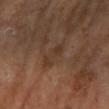  biopsy_status: not biopsied; imaged during a skin examination
  image:
    source: total-body photography crop
    field_of_view_mm: 15
  lighting: cross-polarized
  automated_metrics:
    area_mm2_approx: 4.5
    eccentricity: 0.9
    shape_asymmetry: 0.3
    cielab_L: 33
    cielab_a: 16
    cielab_b: 26
    vs_skin_darker_L: 5.0
    vs_skin_contrast_norm: 5.0
    border_irregularity_0_10: 4.0
    color_variation_0_10: 1.5
  patient:
    sex: female
    age_approx: 65
  site: left lower leg
  lesion_size:
    long_diameter_mm_approx: 3.5